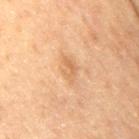diameter: ≈3.5 mm
site: the right thigh
image source: 15 mm crop, total-body photography
illumination: cross-polarized
patient: female, in their mid-50s